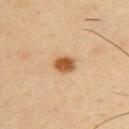notes: catalogued during a skin exam; not biopsied
patient: male, aged 38–42
automated metrics: a lesion color around L≈56 a*≈22 b*≈39 in CIELAB and a lesion–skin lightness drop of about 15; a nevus-likeness score of about 100/100 and a lesion-detection confidence of about 100/100
size: ≈3 mm
site: the upper back
tile lighting: cross-polarized illumination
image source: ~15 mm tile from a whole-body skin photo